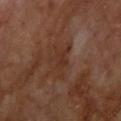lesion_size:
  long_diameter_mm_approx: 4.0
site: back
lighting: cross-polarized
patient:
  sex: male
  age_approx: 70
image:
  source: total-body photography crop
  field_of_view_mm: 15